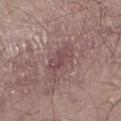Impression: The lesion was photographed on a routine skin check and not biopsied; there is no pathology result. Acquisition and patient details: Approximately 5 mm at its widest. This image is a 15 mm lesion crop taken from a total-body photograph. On the leg. Automated image analysis of the tile measured a shape eccentricity near 0.9 and two-axis asymmetry of about 0.25. The analysis additionally found an average lesion color of about L≈47 a*≈19 b*≈16 (CIELAB), about 8 CIELAB-L* units darker than the surrounding skin, and a lesion-to-skin contrast of about 6 (normalized; higher = more distinct). The software also gave border irregularity of about 3.5 on a 0–10 scale, a color-variation rating of about 3/10, and radial color variation of about 1. The patient is a male aged 58 to 62. Captured under white-light illumination.